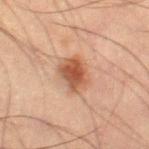The lesion was photographed on a routine skin check and not biopsied; there is no pathology result.
Cropped from a whole-body photographic skin survey; the tile spans about 15 mm.
The subject is a male about 55 years old.
On the left thigh.